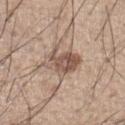The lesion was photographed on a routine skin check and not biopsied; there is no pathology result. Automated image analysis of the tile measured an average lesion color of about L≈54 a*≈17 b*≈25 (CIELAB), a lesion–skin lightness drop of about 12, and a normalized border contrast of about 8.5. The software also gave a classifier nevus-likeness of about 75/100 and a detector confidence of about 100 out of 100 that the crop contains a lesion. This is a white-light tile. A lesion tile, about 15 mm wide, cut from a 3D total-body photograph. The lesion is located on the left lower leg. The patient is a male roughly 35 years of age.